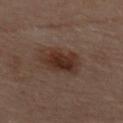{"biopsy_status": "not biopsied; imaged during a skin examination", "lighting": "cross-polarized", "patient": {"sex": "female", "age_approx": 55}, "image": {"source": "total-body photography crop", "field_of_view_mm": 15}, "site": "upper back", "lesion_size": {"long_diameter_mm_approx": 5.0}}